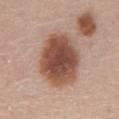workup: total-body-photography surveillance lesion; no biopsy
imaging modality: ~15 mm crop, total-body skin-cancer survey
tile lighting: white-light
location: the abdomen
lesion size: ≈7 mm
patient: male, aged 58 to 62